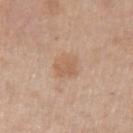Imaged during a routine full-body skin examination; the lesion was not biopsied and no histopathology is available. Measured at roughly 3.5 mm in maximum diameter. The patient is a male in their 60s. On the arm. This is a white-light tile. A close-up tile cropped from a whole-body skin photograph, about 15 mm across.